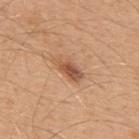No biopsy was performed on this lesion — it was imaged during a full skin examination and was not determined to be concerning.
A male patient, roughly 50 years of age.
Cropped from a total-body skin-imaging series; the visible field is about 15 mm.
The lesion is on the mid back.
The lesion-visualizer software estimated a lesion area of about 5.5 mm², an eccentricity of roughly 0.9, and two-axis asymmetry of about 0.25.
Longest diameter approximately 3.5 mm.